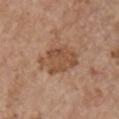Image and clinical context:
This is a white-light tile. A close-up tile cropped from a whole-body skin photograph, about 15 mm across. A female subject, roughly 65 years of age. Longest diameter approximately 5 mm. On the arm.